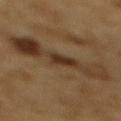Part of a total-body skin-imaging series; this lesion was reviewed on a skin check and was not flagged for biopsy. The tile uses cross-polarized illumination. The total-body-photography lesion software estimated a lesion area of about 36 mm², an eccentricity of roughly 0.9, and two-axis asymmetry of about 0.35. It also reported a lesion–skin lightness drop of about 7. The subject is a male approximately 85 years of age or older. Cropped from a whole-body photographic skin survey; the tile spans about 15 mm. From the mid back. Longest diameter approximately 10.5 mm.